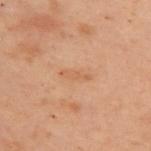workup: imaged on a skin check; not biopsied
illumination: cross-polarized illumination
patient: female, roughly 55 years of age
automated lesion analysis: a lesion area of about 3 mm², an eccentricity of roughly 0.95, and two-axis asymmetry of about 0.35; a lesion–skin lightness drop of about 5; a border-irregularity rating of about 4.5/10 and a color-variation rating of about 0/10; an automated nevus-likeness rating near 0 out of 100 and lesion-presence confidence of about 100/100
image: 15 mm crop, total-body photography
anatomic site: the upper back
size: ≈3.5 mm